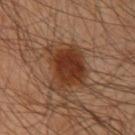workup: total-body-photography surveillance lesion; no biopsy | diameter: ≈5.5 mm | site: the left upper arm | subject: male, aged 43 to 47 | image source: ~15 mm tile from a whole-body skin photo | tile lighting: cross-polarized.